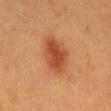workup: no biopsy performed (imaged during a skin exam)
tile lighting: cross-polarized illumination
acquisition: ~15 mm crop, total-body skin-cancer survey
lesion diameter: ~5 mm (longest diameter)
location: the back
subject: female, about 50 years old